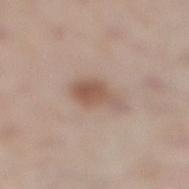Q: Was a biopsy performed?
A: imaged on a skin check; not biopsied
Q: Lesion location?
A: the leg
Q: Lesion size?
A: ~4 mm (longest diameter)
Q: Who is the patient?
A: male, aged 58 to 62
Q: How was this image acquired?
A: total-body-photography crop, ~15 mm field of view
Q: What did automated image analysis measure?
A: a lesion color around L≈55 a*≈17 b*≈26 in CIELAB, roughly 10 lightness units darker than nearby skin, and a normalized border contrast of about 7.5; a classifier nevus-likeness of about 85/100 and lesion-presence confidence of about 100/100